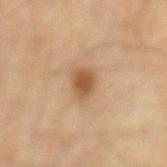Located on the mid back. Cropped from a whole-body photographic skin survey; the tile spans about 15 mm. The patient is a male aged around 85 or older.